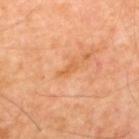Recorded during total-body skin imaging; not selected for excision or biopsy. A roughly 15 mm field-of-view crop from a total-body skin photograph. From the back. A male subject, approximately 55 years of age.